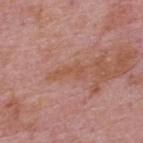{"biopsy_status": "not biopsied; imaged during a skin examination"}